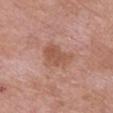Assessment:
Captured during whole-body skin photography for melanoma surveillance; the lesion was not biopsied.
Acquisition and patient details:
This image is a 15 mm lesion crop taken from a total-body photograph. The patient is a female aged 68–72. Measured at roughly 4.5 mm in maximum diameter. Imaged with white-light lighting. From the chest. The total-body-photography lesion software estimated a mean CIELAB color near L≈54 a*≈23 b*≈29 and roughly 8 lightness units darker than nearby skin. And it measured a classifier nevus-likeness of about 0/100 and a detector confidence of about 100 out of 100 that the crop contains a lesion.